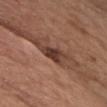Assessment: Imaged during a routine full-body skin examination; the lesion was not biopsied and no histopathology is available. Image and clinical context: From the chest. Cropped from a whole-body photographic skin survey; the tile spans about 15 mm. A male subject in their mid- to late 70s.